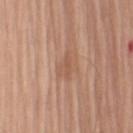Impression: The lesion was photographed on a routine skin check and not biopsied; there is no pathology result. Clinical summary: The patient is a male aged 73–77. About 3 mm across. From the left upper arm. A roughly 15 mm field-of-view crop from a total-body skin photograph. Imaged with white-light lighting.